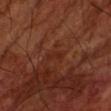Imaged during a routine full-body skin examination; the lesion was not biopsied and no histopathology is available. This image is a 15 mm lesion crop taken from a total-body photograph. The total-body-photography lesion software estimated an area of roughly 2 mm², an eccentricity of roughly 0.95, and a symmetry-axis asymmetry near 0.45. And it measured an average lesion color of about L≈25 a*≈24 b*≈28 (CIELAB), a lesion–skin lightness drop of about 4, and a lesion-to-skin contrast of about 4.5 (normalized; higher = more distinct). A male subject, aged 68 to 72. The lesion is located on the arm. Approximately 2.5 mm at its widest.